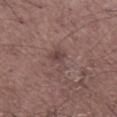Part of a total-body skin-imaging series; this lesion was reviewed on a skin check and was not flagged for biopsy.
A male subject, aged approximately 70.
The tile uses white-light illumination.
This image is a 15 mm lesion crop taken from a total-body photograph.
Longest diameter approximately 3 mm.
The lesion is located on the right lower leg.
The total-body-photography lesion software estimated an area of roughly 4 mm² and an eccentricity of roughly 0.8.